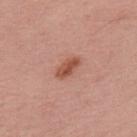notes — total-body-photography surveillance lesion; no biopsy.